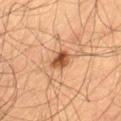Impression: No biopsy was performed on this lesion — it was imaged during a full skin examination and was not determined to be concerning. Image and clinical context: The patient is a male in their mid- to late 60s. A region of skin cropped from a whole-body photographic capture, roughly 15 mm wide. Captured under cross-polarized illumination. Located on the left thigh. Longest diameter approximately 2.5 mm. An algorithmic analysis of the crop reported an area of roughly 4 mm² and an outline eccentricity of about 0.75 (0 = round, 1 = elongated). The analysis additionally found an average lesion color of about L≈43 a*≈21 b*≈32 (CIELAB) and about 14 CIELAB-L* units darker than the surrounding skin. The analysis additionally found border irregularity of about 3 on a 0–10 scale and radial color variation of about 1.5.